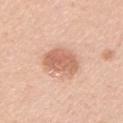Impression:
Part of a total-body skin-imaging series; this lesion was reviewed on a skin check and was not flagged for biopsy.
Context:
Measured at roughly 4 mm in maximum diameter. A roughly 15 mm field-of-view crop from a total-body skin photograph. From the left upper arm. Automated image analysis of the tile measured a footprint of about 11 mm², an outline eccentricity of about 0.5 (0 = round, 1 = elongated), and a shape-asymmetry score of about 0.2 (0 = symmetric). And it measured a mean CIELAB color near L≈64 a*≈24 b*≈32, a lesion–skin lightness drop of about 12, and a normalized border contrast of about 7.5. The analysis additionally found a color-variation rating of about 3/10 and peripheral color asymmetry of about 1. A female subject in their 40s.